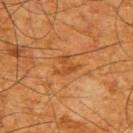Notes:
– notes — total-body-photography surveillance lesion; no biopsy
– lighting — cross-polarized illumination
– subject — male, aged 63 to 67
– body site — the upper back
– lesion size — ~3 mm (longest diameter)
– acquisition — 15 mm crop, total-body photography